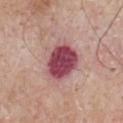No biopsy was performed on this lesion — it was imaged during a full skin examination and was not determined to be concerning.
From the chest.
A male subject, approximately 65 years of age.
An algorithmic analysis of the crop reported an average lesion color of about L≈48 a*≈30 b*≈17 (CIELAB), roughly 18 lightness units darker than nearby skin, and a normalized lesion–skin contrast near 13. The analysis additionally found internal color variation of about 5 on a 0–10 scale and a peripheral color-asymmetry measure near 1.5. The software also gave a nevus-likeness score of about 5/100 and lesion-presence confidence of about 100/100.
Approximately 5 mm at its widest.
A region of skin cropped from a whole-body photographic capture, roughly 15 mm wide.
Captured under white-light illumination.